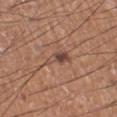tile lighting: white-light
body site: the left lower leg
subject: male, about 55 years old
lesion size: about 3.5 mm
imaging modality: ~15 mm tile from a whole-body skin photo